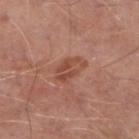* follow-up — no biopsy performed (imaged during a skin exam)
* site — the right lower leg
* patient — male, aged around 65
* image source — total-body-photography crop, ~15 mm field of view
* illumination — white-light illumination